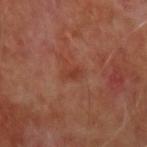Findings:
* follow-up: imaged on a skin check; not biopsied
* image: 15 mm crop, total-body photography
* lesion size: ≈2 mm
* tile lighting: cross-polarized illumination
* patient: male, in their 50s
* site: the left forearm